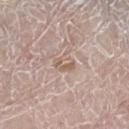No biopsy was performed on this lesion — it was imaged during a full skin examination and was not determined to be concerning. Measured at roughly 2.5 mm in maximum diameter. The tile uses white-light illumination. An algorithmic analysis of the crop reported a color-variation rating of about 4/10 and a peripheral color-asymmetry measure near 1.5. The analysis additionally found lesion-presence confidence of about 60/100. A male patient approximately 80 years of age. Located on the right lower leg. A roughly 15 mm field-of-view crop from a total-body skin photograph.